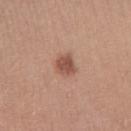Q: Was this lesion biopsied?
A: total-body-photography surveillance lesion; no biopsy
Q: Lesion size?
A: about 2.5 mm
Q: Where on the body is the lesion?
A: the right lower leg
Q: What is the imaging modality?
A: 15 mm crop, total-body photography
Q: What lighting was used for the tile?
A: white-light
Q: What are the patient's age and sex?
A: female, in their mid- to late 40s